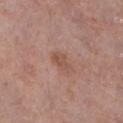Clinical impression: Part of a total-body skin-imaging series; this lesion was reviewed on a skin check and was not flagged for biopsy. Clinical summary: From the right lower leg. Imaged with white-light lighting. The subject is a male aged 53–57. A roughly 15 mm field-of-view crop from a total-body skin photograph. Longest diameter approximately 2.5 mm.